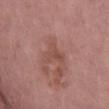Part of a total-body skin-imaging series; this lesion was reviewed on a skin check and was not flagged for biopsy. A female subject about 40 years old. Measured at roughly 3.5 mm in maximum diameter. Imaged with white-light lighting. From the right thigh. A 15 mm crop from a total-body photograph taken for skin-cancer surveillance. The total-body-photography lesion software estimated a mean CIELAB color near L≈48 a*≈23 b*≈24, a lesion–skin lightness drop of about 7, and a normalized border contrast of about 5.5. The software also gave a within-lesion color-variation index near 1.5/10 and radial color variation of about 0.5. It also reported an automated nevus-likeness rating near 0 out of 100.